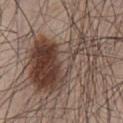Imaged during a routine full-body skin examination; the lesion was not biopsied and no histopathology is available. The patient is a male aged approximately 50. Cropped from a whole-body photographic skin survey; the tile spans about 15 mm. This is a white-light tile. From the front of the torso.